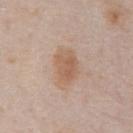Q: What is the anatomic site?
A: the front of the torso
Q: What is the imaging modality?
A: ~15 mm tile from a whole-body skin photo
Q: Illumination type?
A: white-light
Q: Patient demographics?
A: male, approximately 75 years of age
Q: What did automated image analysis measure?
A: a footprint of about 9 mm² and a symmetry-axis asymmetry near 0.15; a lesion color around L≈59 a*≈18 b*≈30 in CIELAB, roughly 9 lightness units darker than nearby skin, and a normalized border contrast of about 7; border irregularity of about 1.5 on a 0–10 scale, a color-variation rating of about 3.5/10, and a peripheral color-asymmetry measure near 1